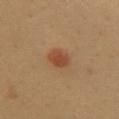This image is a 15 mm lesion crop taken from a total-body photograph. The lesion is on the front of the torso. A female patient, about 50 years old.